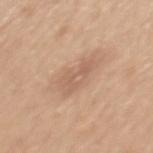{"biopsy_status": "not biopsied; imaged during a skin examination", "patient": {"sex": "male", "age_approx": 50}, "lighting": "white-light", "image": {"source": "total-body photography crop", "field_of_view_mm": 15}, "lesion_size": {"long_diameter_mm_approx": 4.5}, "site": "mid back", "automated_metrics": {"area_mm2_approx": 8.0, "eccentricity": 0.85, "shape_asymmetry": 0.35, "color_variation_0_10": 2.5, "peripheral_color_asymmetry": 1.0, "nevus_likeness_0_100": 0, "lesion_detection_confidence_0_100": 100}}